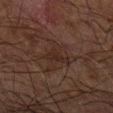Case summary:
• follow-up — no biopsy performed (imaged during a skin exam)